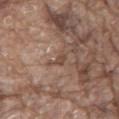Assessment:
Imaged during a routine full-body skin examination; the lesion was not biopsied and no histopathology is available.
Image and clinical context:
The lesion's longest dimension is about 3 mm. The lesion is located on the mid back. Captured under white-light illumination. A 15 mm close-up extracted from a 3D total-body photography capture. A male subject aged 78 to 82. The lesion-visualizer software estimated a footprint of about 3 mm² and an eccentricity of roughly 0.85. The software also gave a mean CIELAB color near L≈47 a*≈17 b*≈26, about 8 CIELAB-L* units darker than the surrounding skin, and a normalized lesion–skin contrast near 6.5.